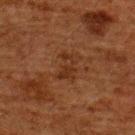Notes:
– follow-up · no biopsy performed (imaged during a skin exam)
– tile lighting · cross-polarized illumination
– patient · male, aged around 60
– lesion diameter · ~3.5 mm (longest diameter)
– image · ~15 mm crop, total-body skin-cancer survey
– site · the back